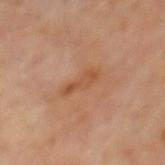biopsy_status: not biopsied; imaged during a skin examination
automated_metrics:
  area_mm2_approx: 5.0
  eccentricity: 0.95
  shape_asymmetry: 0.35
  nevus_likeness_0_100: 0
site: mid back
image:
  source: total-body photography crop
  field_of_view_mm: 15
lighting: cross-polarized
patient:
  sex: male
  age_approx: 70